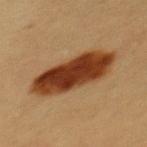This lesion was catalogued during total-body skin photography and was not selected for biopsy.
A close-up tile cropped from a whole-body skin photograph, about 15 mm across.
The recorded lesion diameter is about 8.5 mm.
The lesion-visualizer software estimated a lesion color around L≈33 a*≈23 b*≈32 in CIELAB and a lesion-to-skin contrast of about 14.5 (normalized; higher = more distinct).
On the mid back.
A female patient in their 20s.